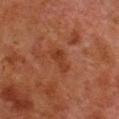The lesion was photographed on a routine skin check and not biopsied; there is no pathology result. A male subject aged 78 to 82. On the leg. Approximately 3.5 mm at its widest. This is a cross-polarized tile. A region of skin cropped from a whole-body photographic capture, roughly 15 mm wide.